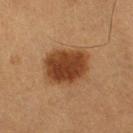Impression:
This lesion was catalogued during total-body skin photography and was not selected for biopsy.
Clinical summary:
A 15 mm crop from a total-body photograph taken for skin-cancer surveillance. Imaged with cross-polarized lighting. Automated image analysis of the tile measured a footprint of about 18 mm², an eccentricity of roughly 0.6, and two-axis asymmetry of about 0.15. And it measured an average lesion color of about L≈36 a*≈20 b*≈32 (CIELAB) and roughly 13 lightness units darker than nearby skin. The software also gave border irregularity of about 2 on a 0–10 scale, a color-variation rating of about 3.5/10, and radial color variation of about 1. The analysis additionally found a lesion-detection confidence of about 100/100. Located on the right lower leg. Approximately 5 mm at its widest. The subject is a male aged around 55.